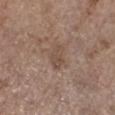Imaged during a routine full-body skin examination; the lesion was not biopsied and no histopathology is available.
A 15 mm crop from a total-body photograph taken for skin-cancer surveillance.
Captured under white-light illumination.
Located on the leg.
Approximately 3 mm at its widest.
A female patient, in their mid- to late 60s.